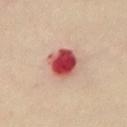<lesion>
  <biopsy_status>not biopsied; imaged during a skin examination</biopsy_status>
  <automated_metrics>
    <area_mm2_approx>10.0</area_mm2_approx>
    <eccentricity>0.45</eccentricity>
    <nevus_likeness_0_100>0</nevus_likeness_0_100>
    <lesion_detection_confidence_0_100>100</lesion_detection_confidence_0_100>
  </automated_metrics>
  <site>chest</site>
  <patient>
    <sex>female</sex>
    <age_approx>55</age_approx>
  </patient>
  <image>
    <source>total-body photography crop</source>
    <field_of_view_mm>15</field_of_view_mm>
  </image>
  <lesion_size>
    <long_diameter_mm_approx>4.0</long_diameter_mm_approx>
  </lesion_size>
  <lighting>cross-polarized</lighting>
</lesion>